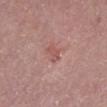  biopsy_status: not biopsied; imaged during a skin examination
  site: left lower leg
  lighting: white-light
  image:
    source: total-body photography crop
    field_of_view_mm: 15
  patient:
    sex: male
    age_approx: 45
  lesion_size:
    long_diameter_mm_approx: 3.0